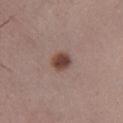Clinical impression:
The lesion was photographed on a routine skin check and not biopsied; there is no pathology result.
Image and clinical context:
The lesion-visualizer software estimated a mean CIELAB color near L≈43 a*≈19 b*≈23, a lesion–skin lightness drop of about 14, and a normalized lesion–skin contrast near 10.5. It also reported border irregularity of about 1.5 on a 0–10 scale, a within-lesion color-variation index near 3/10, and peripheral color asymmetry of about 1. On the right lower leg. Longest diameter approximately 2.5 mm. This image is a 15 mm lesion crop taken from a total-body photograph. The patient is a male roughly 55 years of age. The tile uses white-light illumination.